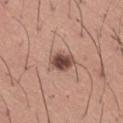Case summary:
• workup: no biopsy performed (imaged during a skin exam)
• lighting: white-light
• size: ≈3.5 mm
• subject: male, aged approximately 45
• anatomic site: the right thigh
• image: ~15 mm crop, total-body skin-cancer survey